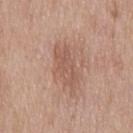* workup — total-body-photography surveillance lesion; no biopsy
* automated lesion analysis — an area of roughly 8.5 mm², an eccentricity of roughly 0.9, and a shape-asymmetry score of about 0.25 (0 = symmetric)
* location — the chest
* illumination — white-light illumination
* size — about 5 mm
* acquisition — 15 mm crop, total-body photography
* subject — male, roughly 50 years of age